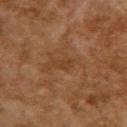Q: Is there a histopathology result?
A: total-body-photography surveillance lesion; no biopsy
Q: How was this image acquired?
A: 15 mm crop, total-body photography
Q: How was the tile lit?
A: cross-polarized
Q: Lesion location?
A: the upper back
Q: How large is the lesion?
A: about 2.5 mm
Q: Who is the patient?
A: female, aged 58 to 62